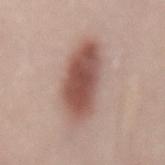This lesion was catalogued during total-body skin photography and was not selected for biopsy.
On the back.
Measured at roughly 8 mm in maximum diameter.
A region of skin cropped from a whole-body photographic capture, roughly 15 mm wide.
A female subject about 50 years old.
This is a white-light tile.